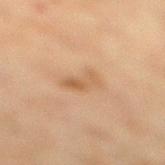Case summary:
– biopsy status: catalogued during a skin exam; not biopsied
– illumination: cross-polarized
– patient: female, aged 38–42
– site: the right lower leg
– automated lesion analysis: an automated nevus-likeness rating near 5 out of 100 and a lesion-detection confidence of about 100/100
– size: ~4 mm (longest diameter)
– acquisition: ~15 mm crop, total-body skin-cancer survey